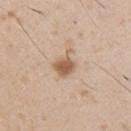<lesion>
  <image>
    <source>total-body photography crop</source>
    <field_of_view_mm>15</field_of_view_mm>
  </image>
  <lesion_size>
    <long_diameter_mm_approx>3.5</long_diameter_mm_approx>
  </lesion_size>
  <patient>
    <sex>male</sex>
    <age_approx>50</age_approx>
  </patient>
  <automated_metrics>
    <nevus_likeness_0_100>85</nevus_likeness_0_100>
    <lesion_detection_confidence_0_100>100</lesion_detection_confidence_0_100>
  </automated_metrics>
  <site>right upper arm</site>
</lesion>